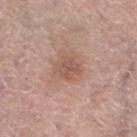biopsy_status: not biopsied; imaged during a skin examination
lesion_size:
  long_diameter_mm_approx: 3.0
patient:
  sex: female
  age_approx: 65
image:
  source: total-body photography crop
  field_of_view_mm: 15
site: left lower leg